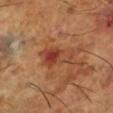Q: Is there a histopathology result?
A: catalogued during a skin exam; not biopsied
Q: What are the patient's age and sex?
A: male, aged around 65
Q: Where on the body is the lesion?
A: the left lower leg
Q: How was this image acquired?
A: ~15 mm crop, total-body skin-cancer survey
Q: Illumination type?
A: cross-polarized